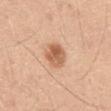<tbp_lesion>
  <biopsy_status>not biopsied; imaged during a skin examination</biopsy_status>
  <patient>
    <sex>male</sex>
    <age_approx>50</age_approx>
  </patient>
  <image>
    <source>total-body photography crop</source>
    <field_of_view_mm>15</field_of_view_mm>
  </image>
  <site>front of the torso</site>
  <lesion_size>
    <long_diameter_mm_approx>3.0</long_diameter_mm_approx>
  </lesion_size>
  <lighting>white-light</lighting>
</tbp_lesion>